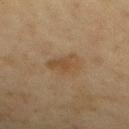The lesion was tiled from a total-body skin photograph and was not biopsied. On the left lower leg. The tile uses cross-polarized illumination. Cropped from a whole-body photographic skin survey; the tile spans about 15 mm. The total-body-photography lesion software estimated a lesion area of about 7 mm² and a shape eccentricity near 0.7. And it measured a mean CIELAB color near L≈39 a*≈13 b*≈29, about 6 CIELAB-L* units darker than the surrounding skin, and a lesion-to-skin contrast of about 6 (normalized; higher = more distinct). It also reported border irregularity of about 2.5 on a 0–10 scale and internal color variation of about 3 on a 0–10 scale. The analysis additionally found a classifier nevus-likeness of about 0/100. The recorded lesion diameter is about 3.5 mm. A female subject aged approximately 50.